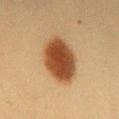Impression:
Imaged during a routine full-body skin examination; the lesion was not biopsied and no histopathology is available.
Acquisition and patient details:
A close-up tile cropped from a whole-body skin photograph, about 15 mm across. Longest diameter approximately 6 mm. A female subject, aged approximately 40. Captured under cross-polarized illumination. The lesion is on the mid back.